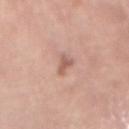The lesion was photographed on a routine skin check and not biopsied; there is no pathology result. Cropped from a total-body skin-imaging series; the visible field is about 15 mm. A female patient, approximately 65 years of age. The lesion is on the abdomen.